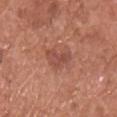diameter = ≈3.5 mm | patient = male, aged 73–77 | image-analysis metrics = an automated nevus-likeness rating near 5 out of 100 and a lesion-detection confidence of about 100/100 | site = the chest | imaging modality = total-body-photography crop, ~15 mm field of view | illumination = white-light illumination.